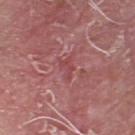* biopsy status — no biopsy performed (imaged during a skin exam)
* imaging modality — ~15 mm crop, total-body skin-cancer survey
* patient — male, about 65 years old
* tile lighting — white-light illumination
* lesion size — ≈2.5 mm
* location — the head or neck